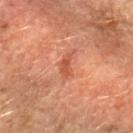| field | value |
|---|---|
| patient | male, in their mid- to late 60s |
| image-analysis metrics | an area of roughly 3 mm², a shape eccentricity near 0.9, and a symmetry-axis asymmetry near 0.4; border irregularity of about 4.5 on a 0–10 scale and a peripheral color-asymmetry measure near 0 |
| size | about 3 mm |
| image | ~15 mm tile from a whole-body skin photo |
| body site | the left forearm |
| tile lighting | cross-polarized |